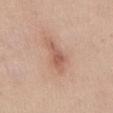{"biopsy_status": "not biopsied; imaged during a skin examination", "lesion_size": {"long_diameter_mm_approx": 4.0}, "site": "front of the torso", "image": {"source": "total-body photography crop", "field_of_view_mm": 15}, "patient": {"sex": "female", "age_approx": 45}}